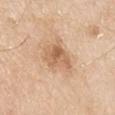Q: Was a biopsy performed?
A: imaged on a skin check; not biopsied
Q: What is the lesion's diameter?
A: ≈4 mm
Q: Automated lesion metrics?
A: an automated nevus-likeness rating near 10 out of 100
Q: Patient demographics?
A: male, approximately 80 years of age
Q: Where on the body is the lesion?
A: the left upper arm
Q: How was this image acquired?
A: 15 mm crop, total-body photography